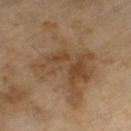Recorded during total-body skin imaging; not selected for excision or biopsy.
This is a cross-polarized tile.
A male patient, about 65 years old.
Approximately 9.5 mm at its widest.
This image is a 15 mm lesion crop taken from a total-body photograph.